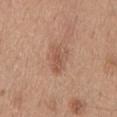The lesion was tiled from a total-body skin photograph and was not biopsied. The total-body-photography lesion software estimated an area of roughly 7 mm², an outline eccentricity of about 0.85 (0 = round, 1 = elongated), and two-axis asymmetry of about 0.3. And it measured a mean CIELAB color near L≈55 a*≈20 b*≈30, about 8 CIELAB-L* units darker than the surrounding skin, and a lesion-to-skin contrast of about 6 (normalized; higher = more distinct). It also reported border irregularity of about 3.5 on a 0–10 scale, a color-variation rating of about 4/10, and a peripheral color-asymmetry measure near 1.5. The software also gave an automated nevus-likeness rating near 5 out of 100 and a lesion-detection confidence of about 100/100. The patient is a male aged 28 to 32. The lesion is located on the back. The tile uses white-light illumination. Approximately 4 mm at its widest. A region of skin cropped from a whole-body photographic capture, roughly 15 mm wide.